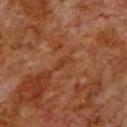Impression:
Imaged during a routine full-body skin examination; the lesion was not biopsied and no histopathology is available.
Acquisition and patient details:
The subject is a male about 80 years old. On the back. Automated tile analysis of the lesion measured a lesion color around L≈29 a*≈20 b*≈28 in CIELAB, about 4 CIELAB-L* units darker than the surrounding skin, and a lesion-to-skin contrast of about 5 (normalized; higher = more distinct). The analysis additionally found border irregularity of about 3 on a 0–10 scale, a within-lesion color-variation index near 0.5/10, and peripheral color asymmetry of about 0. The analysis additionally found a classifier nevus-likeness of about 0/100 and a lesion-detection confidence of about 90/100. A close-up tile cropped from a whole-body skin photograph, about 15 mm across. The recorded lesion diameter is about 2.5 mm. The tile uses cross-polarized illumination.